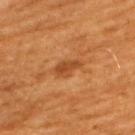Part of a total-body skin-imaging series; this lesion was reviewed on a skin check and was not flagged for biopsy.
A close-up tile cropped from a whole-body skin photograph, about 15 mm across.
The patient is a male aged around 60.
On the upper back.